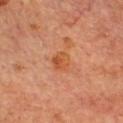notes: total-body-photography surveillance lesion; no biopsy
subject: male, aged 68–72
lighting: cross-polarized
image source: ~15 mm tile from a whole-body skin photo
lesion diameter: ~2.5 mm (longest diameter)
automated metrics: an area of roughly 5 mm² and a shape eccentricity near 0.6; a border-irregularity rating of about 1.5/10 and internal color variation of about 4 on a 0–10 scale
location: the chest